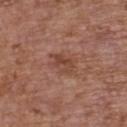Findings:
* biopsy status · catalogued during a skin exam; not biopsied
* subject · female, in their mid-60s
* lighting · white-light illumination
* lesion diameter · about 3 mm
* body site · the upper back
* imaging modality · ~15 mm crop, total-body skin-cancer survey